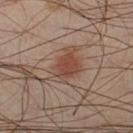workup = total-body-photography surveillance lesion; no biopsy | image source = ~15 mm tile from a whole-body skin photo | subject = male, in their 40s | illumination = cross-polarized illumination | location = the right thigh.